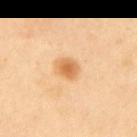This image is a 15 mm lesion crop taken from a total-body photograph.
The lesion's longest dimension is about 2.5 mm.
A female patient aged around 40.
An algorithmic analysis of the crop reported a nevus-likeness score of about 95/100 and a lesion-detection confidence of about 100/100.
The tile uses cross-polarized illumination.
From the upper back.